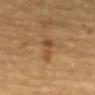workup: imaged on a skin check; not biopsied | size: about 4 mm | illumination: cross-polarized | patient: male, aged approximately 85 or older | imaging modality: total-body-photography crop, ~15 mm field of view | automated lesion analysis: an eccentricity of roughly 0.9; an average lesion color of about L≈41 a*≈17 b*≈33 (CIELAB) | site: the mid back.